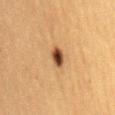| feature | finding |
|---|---|
| biopsy status | catalogued during a skin exam; not biopsied |
| site | the mid back |
| diameter | about 3 mm |
| image | 15 mm crop, total-body photography |
| patient | female, approximately 40 years of age |